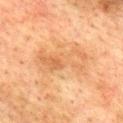Q: Was a biopsy performed?
A: catalogued during a skin exam; not biopsied
Q: What lighting was used for the tile?
A: cross-polarized
Q: Who is the patient?
A: male, roughly 75 years of age
Q: What did automated image analysis measure?
A: a footprint of about 16 mm², an outline eccentricity of about 0.9 (0 = round, 1 = elongated), and two-axis asymmetry of about 0.25; a border-irregularity rating of about 4.5/10, a within-lesion color-variation index near 5.5/10, and peripheral color asymmetry of about 2
Q: Where on the body is the lesion?
A: the upper back
Q: How large is the lesion?
A: ≈7 mm
Q: What is the imaging modality?
A: ~15 mm tile from a whole-body skin photo